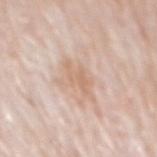Q: Illumination type?
A: white-light
Q: Who is the patient?
A: male, aged around 80
Q: Where on the body is the lesion?
A: the mid back
Q: Lesion size?
A: ~4 mm (longest diameter)
Q: What is the imaging modality?
A: total-body-photography crop, ~15 mm field of view